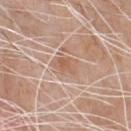<tbp_lesion>
<image>
  <source>total-body photography crop</source>
  <field_of_view_mm>15</field_of_view_mm>
</image>
<patient>
  <sex>male</sex>
  <age_approx>80</age_approx>
</patient>
<site>chest</site>
<lesion_size>
  <long_diameter_mm_approx>3.0</long_diameter_mm_approx>
</lesion_size>
<lighting>white-light</lighting>
<automated_metrics>
  <color_variation_0_10>3.5</color_variation_0_10>
  <peripheral_color_asymmetry>1.0</peripheral_color_asymmetry>
  <nevus_likeness_0_100>0</nevus_likeness_0_100>
  <lesion_detection_confidence_0_100>100</lesion_detection_confidence_0_100>
</automated_metrics>
</tbp_lesion>